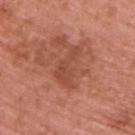The lesion was photographed on a routine skin check and not biopsied; there is no pathology result.